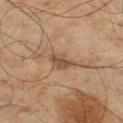Imaged during a routine full-body skin examination; the lesion was not biopsied and no histopathology is available. Approximately 3 mm at its widest. This image is a 15 mm lesion crop taken from a total-body photograph. On the left lower leg. Automated tile analysis of the lesion measured a nevus-likeness score of about 0/100 and a detector confidence of about 100 out of 100 that the crop contains a lesion. This is a cross-polarized tile. A male subject aged 43 to 47.